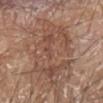automated metrics=internal color variation of about 5 on a 0–10 scale and radial color variation of about 2; an automated nevus-likeness rating near 0 out of 100 and a lesion-detection confidence of about 95/100
image=15 mm crop, total-body photography
illumination=white-light
size=≈9.5 mm
site=the left forearm
subject=male, aged approximately 80